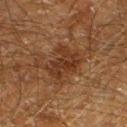The lesion was tiled from a total-body skin photograph and was not biopsied. A male patient, aged 58–62. Cropped from a whole-body photographic skin survey; the tile spans about 15 mm. The lesion is located on the left lower leg. About 4.5 mm across. The lesion-visualizer software estimated an average lesion color of about L≈26 a*≈17 b*≈25 (CIELAB), a lesion–skin lightness drop of about 7, and a normalized border contrast of about 7.5. And it measured border irregularity of about 4.5 on a 0–10 scale and a within-lesion color-variation index near 3/10. The analysis additionally found a classifier nevus-likeness of about 20/100 and a lesion-detection confidence of about 100/100. This is a cross-polarized tile.